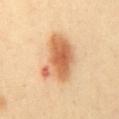Background: The tile uses cross-polarized illumination. A female patient aged approximately 40. The lesion-visualizer software estimated border irregularity of about 3.5 on a 0–10 scale. A 15 mm crop from a total-body photograph taken for skin-cancer surveillance. About 6 mm across. The lesion is on the mid back.